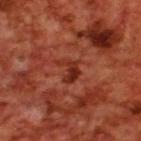Impression: Part of a total-body skin-imaging series; this lesion was reviewed on a skin check and was not flagged for biopsy. Image and clinical context: Approximately 3 mm at its widest. Captured under cross-polarized illumination. A close-up tile cropped from a whole-body skin photograph, about 15 mm across. A male patient aged 68 to 72. The lesion is on the upper back.